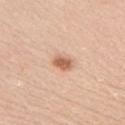Recorded during total-body skin imaging; not selected for excision or biopsy.
Measured at roughly 2.5 mm in maximum diameter.
The lesion-visualizer software estimated a lesion color around L≈62 a*≈23 b*≈33 in CIELAB and a normalized border contrast of about 8.5. It also reported border irregularity of about 2 on a 0–10 scale and a color-variation rating of about 2.5/10. It also reported a classifier nevus-likeness of about 95/100.
A male subject about 40 years old.
A region of skin cropped from a whole-body photographic capture, roughly 15 mm wide.
From the right upper arm.